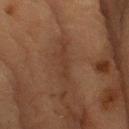Recorded during total-body skin imaging; not selected for excision or biopsy.
Located on the right upper arm.
A male patient, approximately 85 years of age or older.
A 15 mm close-up extracted from a 3D total-body photography capture.